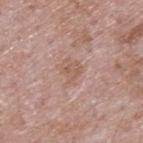Findings:
• follow-up · catalogued during a skin exam; not biopsied
• automated lesion analysis · a lesion color around L≈57 a*≈19 b*≈26 in CIELAB, roughly 7 lightness units darker than nearby skin, and a normalized lesion–skin contrast near 5.5; a border-irregularity rating of about 4.5/10 and a peripheral color-asymmetry measure near 1; lesion-presence confidence of about 100/100
• tile lighting · white-light illumination
• patient · male, about 65 years old
• lesion size · about 3.5 mm
• image source · total-body-photography crop, ~15 mm field of view
• site · the back